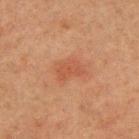<record>
  <lesion_size>
    <long_diameter_mm_approx>3.5</long_diameter_mm_approx>
  </lesion_size>
  <site>back</site>
  <lighting>cross-polarized</lighting>
  <patient>
    <sex>male</sex>
    <age_approx>60</age_approx>
  </patient>
  <image>
    <source>total-body photography crop</source>
    <field_of_view_mm>15</field_of_view_mm>
  </image>
</record>